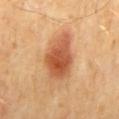Impression:
The lesion was tiled from a total-body skin photograph and was not biopsied.
Clinical summary:
A lesion tile, about 15 mm wide, cut from a 3D total-body photograph. The subject is a male about 55 years old. The lesion is on the mid back.